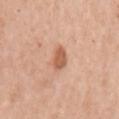| key | value |
|---|---|
| follow-up | imaged on a skin check; not biopsied |
| location | the left upper arm |
| lighting | white-light illumination |
| subject | female, in their mid-60s |
| image | 15 mm crop, total-body photography |